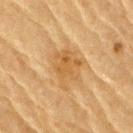diameter — ≈4.5 mm; acquisition — ~15 mm tile from a whole-body skin photo; anatomic site — the right upper arm; patient — male, in their mid-80s or older.